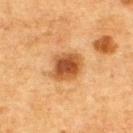{"biopsy_status": "not biopsied; imaged during a skin examination", "patient": {"sex": "male", "age_approx": 75}, "site": "upper back", "lesion_size": {"long_diameter_mm_approx": 4.0}, "image": {"source": "total-body photography crop", "field_of_view_mm": 15}, "lighting": "cross-polarized"}